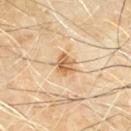follow-up: no biopsy performed (imaged during a skin exam) | anatomic site: the mid back | image-analysis metrics: an area of roughly 5 mm², a shape eccentricity near 0.55, and a symmetry-axis asymmetry near 0.25; about 12 CIELAB-L* units darker than the surrounding skin and a normalized lesion–skin contrast near 8; an automated nevus-likeness rating near 65 out of 100 and a detector confidence of about 100 out of 100 that the crop contains a lesion | acquisition: total-body-photography crop, ~15 mm field of view | patient: male, aged approximately 60 | size: ~2.5 mm (longest diameter).